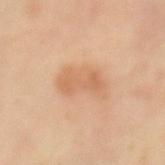biopsy status = no biopsy performed (imaged during a skin exam)
automated lesion analysis = a nevus-likeness score of about 15/100
patient = female, aged around 50
diameter = ~5 mm (longest diameter)
site = the left thigh
imaging modality = 15 mm crop, total-body photography